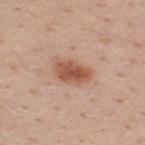Q: Was this lesion biopsied?
A: imaged on a skin check; not biopsied
Q: Where on the body is the lesion?
A: the back
Q: Who is the patient?
A: male, about 40 years old
Q: Lesion size?
A: about 4 mm
Q: How was this image acquired?
A: ~15 mm tile from a whole-body skin photo
Q: Automated lesion metrics?
A: a border-irregularity rating of about 2/10; a nevus-likeness score of about 100/100